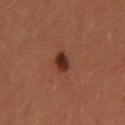workup — catalogued during a skin exam; not biopsied
anatomic site — the left thigh
automated metrics — an area of roughly 3.5 mm² and an eccentricity of roughly 0.85; a lesion color around L≈24 a*≈20 b*≈23 in CIELAB, about 11 CIELAB-L* units darker than the surrounding skin, and a normalized lesion–skin contrast near 11
imaging modality — total-body-photography crop, ~15 mm field of view
lesion size — ≈3 mm
lighting — cross-polarized
subject — female, aged 48 to 52